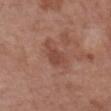Notes:
• biopsy status · imaged on a skin check; not biopsied
• illumination · white-light illumination
• acquisition · ~15 mm crop, total-body skin-cancer survey
• diameter · ~4 mm (longest diameter)
• anatomic site · the chest
• patient · female, roughly 75 years of age